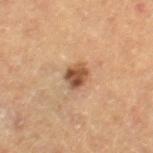<record>
  <biopsy_status>not biopsied; imaged during a skin examination</biopsy_status>
  <patient>
    <sex>female</sex>
    <age_approx>60</age_approx>
  </patient>
  <lesion_size>
    <long_diameter_mm_approx>3.0</long_diameter_mm_approx>
  </lesion_size>
  <site>right thigh</site>
  <automated_metrics>
    <cielab_L>48</cielab_L>
    <cielab_a>20</cielab_a>
    <cielab_b>32</cielab_b>
    <vs_skin_darker_L>14.0</vs_skin_darker_L>
    <vs_skin_contrast_norm>9.5</vs_skin_contrast_norm>
    <border_irregularity_0_10>3.0</border_irregularity_0_10>
    <color_variation_0_10>4.5</color_variation_0_10>
    <peripheral_color_asymmetry>1.5</peripheral_color_asymmetry>
  </automated_metrics>
  <lighting>cross-polarized</lighting>
  <image>
    <source>total-body photography crop</source>
    <field_of_view_mm>15</field_of_view_mm>
  </image>
</record>